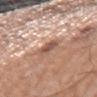Q: Is there a histopathology result?
A: total-body-photography surveillance lesion; no biopsy
Q: Where on the body is the lesion?
A: the arm
Q: What are the patient's age and sex?
A: male, aged around 50
Q: Automated lesion metrics?
A: a lesion area of about 5.5 mm²; an automated nevus-likeness rating near 5 out of 100 and lesion-presence confidence of about 100/100
Q: How large is the lesion?
A: ~3 mm (longest diameter)
Q: What kind of image is this?
A: total-body-photography crop, ~15 mm field of view
Q: Illumination type?
A: white-light illumination